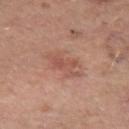subject — female, approximately 40 years of age
lesion size — about 5 mm
image source — total-body-photography crop, ~15 mm field of view
site — the left forearm
illumination — cross-polarized illumination